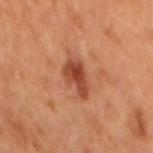Impression: The lesion was photographed on a routine skin check and not biopsied; there is no pathology result. Image and clinical context: Approximately 4.5 mm at its widest. A roughly 15 mm field-of-view crop from a total-body skin photograph. This is a cross-polarized tile. A male patient in their mid- to late 60s. From the mid back.